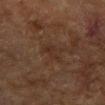Assessment:
The lesion was tiled from a total-body skin photograph and was not biopsied.
Image and clinical context:
Captured under cross-polarized illumination. A 15 mm close-up tile from a total-body photography series done for melanoma screening. A female patient aged 78–82. Approximately 3 mm at its widest. Located on the right forearm.